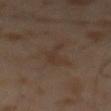| field | value |
|---|---|
| workup | no biopsy performed (imaged during a skin exam) |
| lighting | cross-polarized illumination |
| lesion size | about 5 mm |
| imaging modality | ~15 mm crop, total-body skin-cancer survey |
| subject | male, roughly 65 years of age |